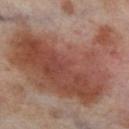<case>
  <biopsy_status>not biopsied; imaged during a skin examination</biopsy_status>
  <image>
    <source>total-body photography crop</source>
    <field_of_view_mm>15</field_of_view_mm>
  </image>
  <patient>
    <sex>female</sex>
    <age_approx>55</age_approx>
  </patient>
  <lesion_size>
    <long_diameter_mm_approx>12.5</long_diameter_mm_approx>
  </lesion_size>
  <lighting>cross-polarized</lighting>
  <site>left thigh</site>
</case>